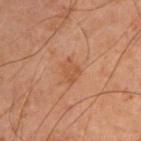Clinical impression:
This lesion was catalogued during total-body skin photography and was not selected for biopsy.
Background:
A male patient aged 48 to 52. The lesion is located on the left upper arm. A region of skin cropped from a whole-body photographic capture, roughly 15 mm wide. Captured under cross-polarized illumination.